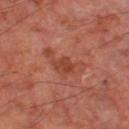{"biopsy_status": "not biopsied; imaged during a skin examination"}